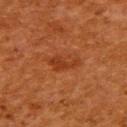Q: Was this lesion biopsied?
A: no biopsy performed (imaged during a skin exam)
Q: What is the imaging modality?
A: 15 mm crop, total-body photography
Q: What is the anatomic site?
A: the upper back
Q: Who is the patient?
A: female, roughly 50 years of age
Q: Illumination type?
A: cross-polarized